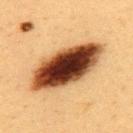Q: Is there a histopathology result?
A: imaged on a skin check; not biopsied
Q: What is the imaging modality?
A: ~15 mm tile from a whole-body skin photo
Q: Where on the body is the lesion?
A: the back
Q: Patient demographics?
A: female, approximately 40 years of age
Q: Automated lesion metrics?
A: a lesion area of about 31 mm² and an eccentricity of roughly 0.9; border irregularity of about 2 on a 0–10 scale, a within-lesion color-variation index near 10/10, and peripheral color asymmetry of about 4; a nevus-likeness score of about 95/100 and a lesion-detection confidence of about 100/100
Q: What is the lesion's diameter?
A: about 9.5 mm
Q: How was the tile lit?
A: cross-polarized illumination